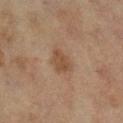Q: Was a biopsy performed?
A: no biopsy performed (imaged during a skin exam)
Q: What is the anatomic site?
A: the leg
Q: How large is the lesion?
A: ≈3.5 mm
Q: What kind of image is this?
A: ~15 mm tile from a whole-body skin photo
Q: Who is the patient?
A: female, approximately 55 years of age